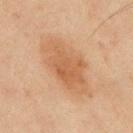This lesion was catalogued during total-body skin photography and was not selected for biopsy. An algorithmic analysis of the crop reported border irregularity of about 2.5 on a 0–10 scale and a within-lesion color-variation index near 3.5/10. The analysis additionally found a lesion-detection confidence of about 100/100. On the upper back. About 8.5 mm across. The tile uses cross-polarized illumination. The subject is a male roughly 45 years of age. A region of skin cropped from a whole-body photographic capture, roughly 15 mm wide.